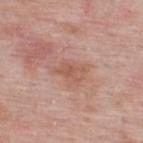Recorded during total-body skin imaging; not selected for excision or biopsy. The lesion is located on the upper back. The total-body-photography lesion software estimated a footprint of about 8 mm², an outline eccentricity of about 0.75 (0 = round, 1 = elongated), and two-axis asymmetry of about 0.4. It also reported about 7 CIELAB-L* units darker than the surrounding skin and a lesion-to-skin contrast of about 5.5 (normalized; higher = more distinct). And it measured a within-lesion color-variation index near 3/10 and a peripheral color-asymmetry measure near 1. Imaged with white-light lighting. A 15 mm close-up extracted from a 3D total-body photography capture. A male subject about 55 years old.